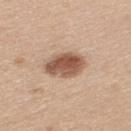Clinical impression: Part of a total-body skin-imaging series; this lesion was reviewed on a skin check and was not flagged for biopsy. Clinical summary: Automated image analysis of the tile measured two-axis asymmetry of about 0.15. It also reported an average lesion color of about L≈54 a*≈20 b*≈29 (CIELAB), a lesion–skin lightness drop of about 16, and a normalized lesion–skin contrast near 10.5. The lesion is located on the upper back. A 15 mm close-up tile from a total-body photography series done for melanoma screening. A female patient, roughly 30 years of age. Longest diameter approximately 4.5 mm.